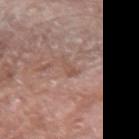The lesion was photographed on a routine skin check and not biopsied; there is no pathology result. A 15 mm close-up extracted from a 3D total-body photography capture. A male subject, about 60 years old. Longest diameter approximately 2.5 mm. The tile uses white-light illumination. The lesion is located on the right upper arm.